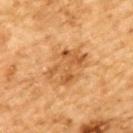Impression:
Imaged during a routine full-body skin examination; the lesion was not biopsied and no histopathology is available.
Background:
Automated image analysis of the tile measured a lesion area of about 18 mm² and an eccentricity of roughly 0.7. The analysis additionally found a normalized border contrast of about 6. A male patient aged approximately 85 or older. The tile uses cross-polarized illumination. A close-up tile cropped from a whole-body skin photograph, about 15 mm across. The lesion is on the back.